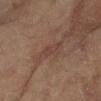workup = total-body-photography surveillance lesion; no biopsy
illumination = cross-polarized
location = the left arm
patient = female, aged around 80
acquisition = 15 mm crop, total-body photography